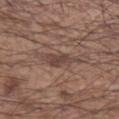This lesion was catalogued during total-body skin photography and was not selected for biopsy.
Imaged with white-light lighting.
The subject is a male aged 53 to 57.
The recorded lesion diameter is about 4.5 mm.
Automated tile analysis of the lesion measured a lesion area of about 6.5 mm² and a shape-asymmetry score of about 0.4 (0 = symmetric). It also reported border irregularity of about 5 on a 0–10 scale, a within-lesion color-variation index near 2/10, and radial color variation of about 0.5. And it measured an automated nevus-likeness rating near 0 out of 100 and a detector confidence of about 70 out of 100 that the crop contains a lesion.
Located on the left forearm.
Cropped from a total-body skin-imaging series; the visible field is about 15 mm.